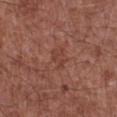No biopsy was performed on this lesion — it was imaged during a full skin examination and was not determined to be concerning. A male patient roughly 65 years of age. The tile uses white-light illumination. Automated image analysis of the tile measured two-axis asymmetry of about 0.4. And it measured an average lesion color of about L≈41 a*≈24 b*≈26 (CIELAB) and about 6 CIELAB-L* units darker than the surrounding skin. The software also gave a lesion-detection confidence of about 100/100. About 2.5 mm across. A 15 mm crop from a total-body photograph taken for skin-cancer surveillance. The lesion is on the right lower leg.